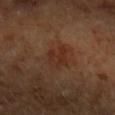No biopsy was performed on this lesion — it was imaged during a full skin examination and was not determined to be concerning. The subject is female. A 15 mm close-up tile from a total-body photography series done for melanoma screening. Located on the right forearm. Automated tile analysis of the lesion measured an area of roughly 6 mm², an eccentricity of roughly 0.7, and a symmetry-axis asymmetry near 0.35. It also reported an automated nevus-likeness rating near 65 out of 100 and a lesion-detection confidence of about 100/100. The recorded lesion diameter is about 3.5 mm.